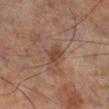Imaged during a routine full-body skin examination; the lesion was not biopsied and no histopathology is available. Approximately 2.5 mm at its widest. This is a cross-polarized tile. A male subject, aged 63 to 67. Located on the leg. A 15 mm crop from a total-body photograph taken for skin-cancer surveillance.